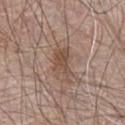{"biopsy_status": "not biopsied; imaged during a skin examination", "image": {"source": "total-body photography crop", "field_of_view_mm": 15}, "patient": {"sex": "male", "age_approx": 65}, "site": "abdomen", "lighting": "white-light", "lesion_size": {"long_diameter_mm_approx": 3.5}}